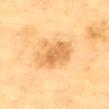Clinical impression: No biopsy was performed on this lesion — it was imaged during a full skin examination and was not determined to be concerning. Background: The tile uses cross-polarized illumination. A female patient, in their 80s. A region of skin cropped from a whole-body photographic capture, roughly 15 mm wide. From the mid back.